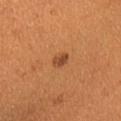Findings:
• notes · no biopsy performed (imaged during a skin exam)
• site · the head or neck
• tile lighting · cross-polarized
• image source · ~15 mm crop, total-body skin-cancer survey
• size · ~2.5 mm (longest diameter)
• subject · female, approximately 40 years of age
• image-analysis metrics · an area of roughly 3 mm², an outline eccentricity of about 0.8 (0 = round, 1 = elongated), and two-axis asymmetry of about 0.25; a lesion color around L≈46 a*≈26 b*≈37 in CIELAB and a normalized lesion–skin contrast near 8; an automated nevus-likeness rating near 100 out of 100 and a detector confidence of about 100 out of 100 that the crop contains a lesion